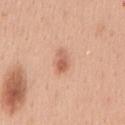automated lesion analysis: a lesion area of about 3.5 mm², a shape eccentricity near 0.85, and a symmetry-axis asymmetry near 0.3; a lesion color around L≈61 a*≈25 b*≈33 in CIELAB, roughly 11 lightness units darker than nearby skin, and a normalized lesion–skin contrast near 7; border irregularity of about 3 on a 0–10 scale, a within-lesion color-variation index near 1.5/10, and peripheral color asymmetry of about 0.5; an automated nevus-likeness rating near 95 out of 100 and a lesion-detection confidence of about 100/100
acquisition: ~15 mm tile from a whole-body skin photo
site: the mid back
illumination: white-light
diameter: ≈3 mm
patient: male, approximately 40 years of age